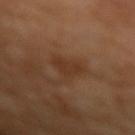Imaged during a routine full-body skin examination; the lesion was not biopsied and no histopathology is available.